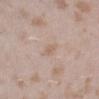<tbp_lesion>
<biopsy_status>not biopsied; imaged during a skin examination</biopsy_status>
<site>left lower leg</site>
<image>
  <source>total-body photography crop</source>
  <field_of_view_mm>15</field_of_view_mm>
</image>
<lighting>white-light</lighting>
<lesion_size>
  <long_diameter_mm_approx>3.0</long_diameter_mm_approx>
</lesion_size>
<patient>
  <sex>female</sex>
  <age_approx>25</age_approx>
</patient>
</tbp_lesion>